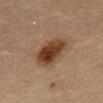Q: Was this lesion biopsied?
A: catalogued during a skin exam; not biopsied
Q: What lighting was used for the tile?
A: cross-polarized illumination
Q: How was this image acquired?
A: 15 mm crop, total-body photography
Q: Who is the patient?
A: male, aged around 85 or older
Q: Lesion location?
A: the chest